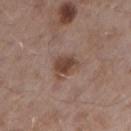| field | value |
|---|---|
| biopsy status | no biopsy performed (imaged during a skin exam) |
| subject | male, aged around 55 |
| lesion diameter | about 3 mm |
| site | the right upper arm |
| tile lighting | white-light illumination |
| image | ~15 mm tile from a whole-body skin photo |